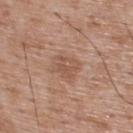| feature | finding |
|---|---|
| image | total-body-photography crop, ~15 mm field of view |
| subject | male, in their 50s |
| TBP lesion metrics | a lesion area of about 5.5 mm², an outline eccentricity of about 0.55 (0 = round, 1 = elongated), and two-axis asymmetry of about 0.25; a lesion color around L≈53 a*≈20 b*≈29 in CIELAB, a lesion–skin lightness drop of about 7, and a normalized lesion–skin contrast near 5.5; a border-irregularity rating of about 2.5/10, internal color variation of about 3 on a 0–10 scale, and radial color variation of about 1; a classifier nevus-likeness of about 0/100 and a detector confidence of about 100 out of 100 that the crop contains a lesion |
| location | the back |
| illumination | white-light illumination |
| lesion diameter | about 3 mm |